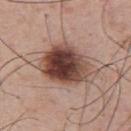<lesion>
<biopsy_status>not biopsied; imaged during a skin examination</biopsy_status>
<site>upper back</site>
<image>
  <source>total-body photography crop</source>
  <field_of_view_mm>15</field_of_view_mm>
</image>
<patient>
  <sex>male</sex>
  <age_approx>55</age_approx>
</patient>
</lesion>